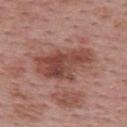Clinical impression: Captured during whole-body skin photography for melanoma surveillance; the lesion was not biopsied. Image and clinical context: Imaged with white-light lighting. A region of skin cropped from a whole-body photographic capture, roughly 15 mm wide. A female subject, in their mid- to late 50s. From the upper back. Longest diameter approximately 7 mm.